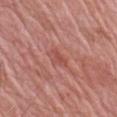{
  "biopsy_status": "not biopsied; imaged during a skin examination",
  "lighting": "white-light",
  "lesion_size": {
    "long_diameter_mm_approx": 2.5
  },
  "site": "right upper arm",
  "image": {
    "source": "total-body photography crop",
    "field_of_view_mm": 15
  },
  "automated_metrics": {
    "vs_skin_darker_L": 7.0,
    "vs_skin_contrast_norm": 5.5
  },
  "patient": {
    "sex": "male",
    "age_approx": 60
  }
}